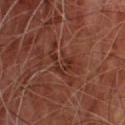Context:
A lesion tile, about 15 mm wide, cut from a 3D total-body photograph. The total-body-photography lesion software estimated an outline eccentricity of about 0.85 (0 = round, 1 = elongated) and a shape-asymmetry score of about 0.65 (0 = symmetric). The software also gave a mean CIELAB color near L≈30 a*≈23 b*≈26 and about 7 CIELAB-L* units darker than the surrounding skin. The software also gave a detector confidence of about 80 out of 100 that the crop contains a lesion. Approximately 5 mm at its widest. This is a cross-polarized tile. A male patient roughly 65 years of age.